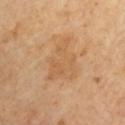Findings:
– follow-up — catalogued during a skin exam; not biopsied
– location — the left arm
– image — 15 mm crop, total-body photography
– subject — male, about 65 years old
– lesion size — about 5.5 mm
– illumination — cross-polarized illumination